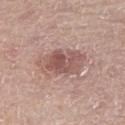Imaged with white-light lighting. The recorded lesion diameter is about 6 mm. Located on the right thigh. This image is a 15 mm lesion crop taken from a total-body photograph. A male subject aged 73–77.